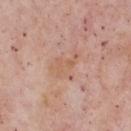Findings:
• follow-up · total-body-photography surveillance lesion; no biopsy
• body site · the chest
• illumination · white-light illumination
• acquisition · total-body-photography crop, ~15 mm field of view
• patient · male, in their mid- to late 50s
• lesion size · ~3.5 mm (longest diameter)